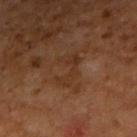Recorded during total-body skin imaging; not selected for excision or biopsy. The total-body-photography lesion software estimated a lesion area of about 7 mm², an eccentricity of roughly 0.85, and two-axis asymmetry of about 0.5. The analysis additionally found a lesion color around L≈30 a*≈18 b*≈28 in CIELAB, a lesion–skin lightness drop of about 5, and a normalized border contrast of about 5. And it measured a classifier nevus-likeness of about 0/100 and a detector confidence of about 95 out of 100 that the crop contains a lesion. A male patient aged 58–62. About 4 mm across. The lesion is on the right upper arm. This is a cross-polarized tile. A 15 mm crop from a total-body photograph taken for skin-cancer surveillance.